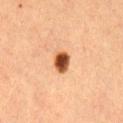{
  "patient": {
    "sex": "female",
    "age_approx": 40
  },
  "image": {
    "source": "total-body photography crop",
    "field_of_view_mm": 15
  },
  "automated_metrics": {
    "area_mm2_approx": 5.0,
    "shape_asymmetry": 0.2,
    "cielab_L": 43,
    "cielab_a": 24,
    "cielab_b": 34,
    "vs_skin_darker_L": 19.0,
    "vs_skin_contrast_norm": 14.0,
    "border_irregularity_0_10": 1.5,
    "color_variation_0_10": 4.5,
    "peripheral_color_asymmetry": 1.0,
    "lesion_detection_confidence_0_100": 100
  },
  "lighting": "cross-polarized",
  "site": "lower back"
}